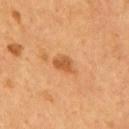{"image": {"source": "total-body photography crop", "field_of_view_mm": 15}, "site": "mid back", "patient": {"sex": "male", "age_approx": 55}}